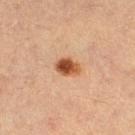The lesion was photographed on a routine skin check and not biopsied; there is no pathology result. A female patient, approximately 30 years of age. Cropped from a total-body skin-imaging series; the visible field is about 15 mm. The lesion is on the right thigh.